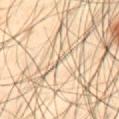Captured during whole-body skin photography for melanoma surveillance; the lesion was not biopsied.
Automated image analysis of the tile measured a shape eccentricity near 0.8 and a symmetry-axis asymmetry near 0.3.
The lesion is located on the abdomen.
Longest diameter approximately 1 mm.
A male patient roughly 40 years of age.
Cropped from a whole-body photographic skin survey; the tile spans about 15 mm.
Captured under cross-polarized illumination.